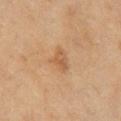Recorded during total-body skin imaging; not selected for excision or biopsy.
The patient is a female aged 58–62.
The total-body-photography lesion software estimated an area of roughly 5 mm², a shape eccentricity near 0.75, and a shape-asymmetry score of about 0.4 (0 = symmetric). It also reported a nevus-likeness score of about 0/100 and a detector confidence of about 100 out of 100 that the crop contains a lesion.
A 15 mm crop from a total-body photograph taken for skin-cancer surveillance.
From the right upper arm.
The tile uses cross-polarized illumination.
About 3.5 mm across.